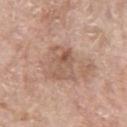biopsy status: total-body-photography surveillance lesion; no biopsy
patient: female, in their mid- to late 70s
imaging modality: ~15 mm tile from a whole-body skin photo
location: the left thigh